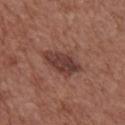Part of a total-body skin-imaging series; this lesion was reviewed on a skin check and was not flagged for biopsy. The lesion is located on the chest. Imaged with white-light lighting. A male subject in their mid- to late 70s. Approximately 5 mm at its widest. A 15 mm close-up extracted from a 3D total-body photography capture.